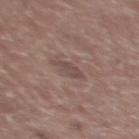{"biopsy_status": "not biopsied; imaged during a skin examination", "patient": {"sex": "male", "age_approx": 60}, "image": {"source": "total-body photography crop", "field_of_view_mm": 15}, "site": "upper back", "lesion_size": {"long_diameter_mm_approx": 3.0}}